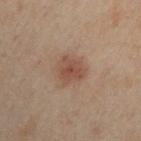Acquisition and patient details:
From the left upper arm. A roughly 15 mm field-of-view crop from a total-body skin photograph. A male patient approximately 50 years of age.